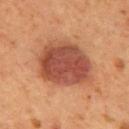The lesion was tiled from a total-body skin photograph and was not biopsied. A male subject in their mid-50s. The lesion is located on the back. A region of skin cropped from a whole-body photographic capture, roughly 15 mm wide.